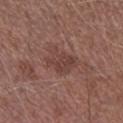Impression: Part of a total-body skin-imaging series; this lesion was reviewed on a skin check and was not flagged for biopsy. Acquisition and patient details: A 15 mm crop from a total-body photograph taken for skin-cancer surveillance. The recorded lesion diameter is about 4 mm. The patient is a male aged approximately 75. The lesion is on the right lower leg. The tile uses white-light illumination.